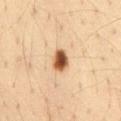Captured during whole-body skin photography for melanoma surveillance; the lesion was not biopsied.
A male subject, in their mid- to late 30s.
On the lower back.
The tile uses cross-polarized illumination.
A roughly 15 mm field-of-view crop from a total-body skin photograph.
The recorded lesion diameter is about 2.5 mm.